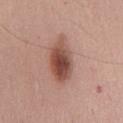Assessment:
The lesion was tiled from a total-body skin photograph and was not biopsied.
Image and clinical context:
The lesion is located on the chest. A lesion tile, about 15 mm wide, cut from a 3D total-body photograph. A male subject aged approximately 65.